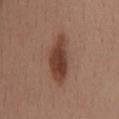Assessment:
Captured during whole-body skin photography for melanoma surveillance; the lesion was not biopsied.
Image and clinical context:
The subject is a female in their mid-50s. A 15 mm crop from a total-body photograph taken for skin-cancer surveillance. The tile uses white-light illumination. The lesion is located on the mid back. Longest diameter approximately 6.5 mm.